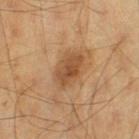biopsy status: catalogued during a skin exam; not biopsied | illumination: cross-polarized illumination | acquisition: ~15 mm crop, total-body skin-cancer survey | size: ~4.5 mm (longest diameter) | TBP lesion metrics: a footprint of about 9.5 mm² and a symmetry-axis asymmetry near 0.2; internal color variation of about 4 on a 0–10 scale and a peripheral color-asymmetry measure near 1; a nevus-likeness score of about 75/100 and lesion-presence confidence of about 100/100 | site: the left thigh | patient: male, aged around 70.